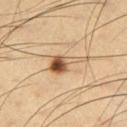Imaged during a routine full-body skin examination; the lesion was not biopsied and no histopathology is available. A roughly 15 mm field-of-view crop from a total-body skin photograph. A male patient, aged 63 to 67. Imaged with cross-polarized lighting. The lesion is on the left thigh. Automated image analysis of the tile measured a mean CIELAB color near L≈56 a*≈18 b*≈35, a lesion–skin lightness drop of about 15, and a normalized lesion–skin contrast near 9.5.